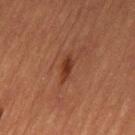notes = no biopsy performed (imaged during a skin exam)
automated lesion analysis = a lesion color around L≈32 a*≈23 b*≈28 in CIELAB and roughly 8 lightness units darker than nearby skin; an automated nevus-likeness rating near 85 out of 100
patient = male, aged 83 or over
lighting = cross-polarized illumination
image = ~15 mm tile from a whole-body skin photo
anatomic site = the left thigh